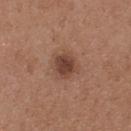Findings:
- notes · no biopsy performed (imaged during a skin exam)
- site · the upper back
- subject · female, approximately 30 years of age
- tile lighting · white-light
- image · total-body-photography crop, ~15 mm field of view
- diameter · ~3 mm (longest diameter)